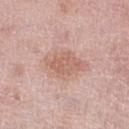Case summary:
– biopsy status — imaged on a skin check; not biopsied
– subject — female, in their 60s
– body site — the right lower leg
– image source — ~15 mm crop, total-body skin-cancer survey